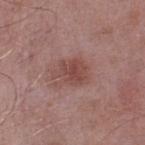Impression: This lesion was catalogued during total-body skin photography and was not selected for biopsy. Image and clinical context: Captured under white-light illumination. A roughly 15 mm field-of-view crop from a total-body skin photograph. An algorithmic analysis of the crop reported a footprint of about 8.5 mm² and a shape-asymmetry score of about 0.3 (0 = symmetric). The analysis additionally found a within-lesion color-variation index near 3/10. The lesion is on the right lower leg. The subject is a male in their mid- to late 50s.